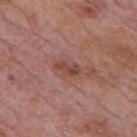Assessment:
Part of a total-body skin-imaging series; this lesion was reviewed on a skin check and was not flagged for biopsy.
Image and clinical context:
The lesion is on the mid back. This is a white-light tile. A 15 mm crop from a total-body photograph taken for skin-cancer surveillance. A male subject aged around 75.